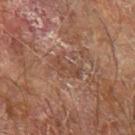The lesion was photographed on a routine skin check and not biopsied; there is no pathology result.
The lesion is on the left forearm.
A male patient aged 68–72.
A region of skin cropped from a whole-body photographic capture, roughly 15 mm wide.
Longest diameter approximately 3.5 mm.